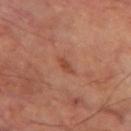biopsy status — catalogued during a skin exam; not biopsied
subject — male, aged approximately 70
tile lighting — cross-polarized
size — ≈2.5 mm
image — ~15 mm tile from a whole-body skin photo
body site — the left thigh